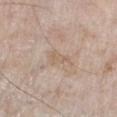Impression:
The lesion was tiled from a total-body skin photograph and was not biopsied.
Clinical summary:
Automated image analysis of the tile measured a footprint of about 3.5 mm², an eccentricity of roughly 0.85, and two-axis asymmetry of about 0.45. It also reported a mean CIELAB color near L≈61 a*≈14 b*≈28 and about 6 CIELAB-L* units darker than the surrounding skin. The lesion is on the right lower leg. A 15 mm close-up extracted from a 3D total-body photography capture. Approximately 3 mm at its widest. A male subject, about 60 years old.